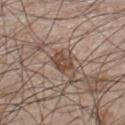Clinical impression: Part of a total-body skin-imaging series; this lesion was reviewed on a skin check and was not flagged for biopsy. Clinical summary: A close-up tile cropped from a whole-body skin photograph, about 15 mm across. This is a white-light tile. Located on the chest. The subject is a male in their 50s. The recorded lesion diameter is about 2.5 mm. The lesion-visualizer software estimated a lesion area of about 4.5 mm², an eccentricity of roughly 0.65, and a symmetry-axis asymmetry near 0.25. The software also gave a lesion color around L≈46 a*≈18 b*≈27 in CIELAB, roughly 10 lightness units darker than nearby skin, and a normalized border contrast of about 8.5. And it measured a border-irregularity index near 2.5/10, a color-variation rating of about 2.5/10, and a peripheral color-asymmetry measure near 1. The analysis additionally found a classifier nevus-likeness of about 80/100 and a lesion-detection confidence of about 85/100.